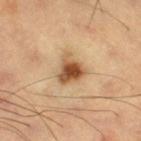Part of a total-body skin-imaging series; this lesion was reviewed on a skin check and was not flagged for biopsy. A 15 mm close-up tile from a total-body photography series done for melanoma screening. About 3.5 mm across. On the right thigh. A male subject aged approximately 60.